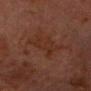Imaged during a routine full-body skin examination; the lesion was not biopsied and no histopathology is available. An algorithmic analysis of the crop reported a lesion color around L≈24 a*≈18 b*≈23 in CIELAB, a lesion–skin lightness drop of about 4, and a normalized lesion–skin contrast near 5.5. The software also gave border irregularity of about 5.5 on a 0–10 scale, a within-lesion color-variation index near 2/10, and radial color variation of about 1. The software also gave a detector confidence of about 95 out of 100 that the crop contains a lesion. Captured under cross-polarized illumination. Measured at roughly 6 mm in maximum diameter. The patient is a male aged around 75. The lesion is on the head or neck. This image is a 15 mm lesion crop taken from a total-body photograph.